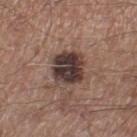Imaged during a routine full-body skin examination; the lesion was not biopsied and no histopathology is available.
This image is a 15 mm lesion crop taken from a total-body photograph.
Imaged with white-light lighting.
The lesion is on the right lower leg.
The patient is a male about 55 years old.
The lesion-visualizer software estimated border irregularity of about 2 on a 0–10 scale, internal color variation of about 5.5 on a 0–10 scale, and radial color variation of about 1.5. The analysis additionally found a lesion-detection confidence of about 100/100.